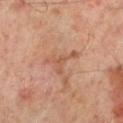Findings:
• follow-up — catalogued during a skin exam; not biopsied
• anatomic site — the left lower leg
• automated metrics — an area of roughly 5 mm², a shape eccentricity near 0.85, and a symmetry-axis asymmetry near 0.75; a lesion color around L≈55 a*≈22 b*≈32 in CIELAB, roughly 7 lightness units darker than nearby skin, and a normalized lesion–skin contrast near 5.5
• lesion diameter — about 4.5 mm
• acquisition — 15 mm crop, total-body photography
• patient — male, approximately 50 years of age